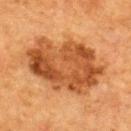The lesion was tiled from a total-body skin photograph and was not biopsied.
A region of skin cropped from a whole-body photographic capture, roughly 15 mm wide.
A female subject aged approximately 55.
The lesion is on the upper back.